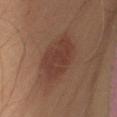Q: Who is the patient?
A: female, aged 58–62
Q: How large is the lesion?
A: ~6.5 mm (longest diameter)
Q: What is the imaging modality?
A: ~15 mm tile from a whole-body skin photo
Q: Lesion location?
A: the right thigh
Q: How was the tile lit?
A: cross-polarized